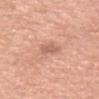notes = catalogued during a skin exam; not biopsied
lesion size = ~2.5 mm (longest diameter)
location = the head or neck
tile lighting = white-light
patient = female, roughly 35 years of age
imaging modality = ~15 mm tile from a whole-body skin photo